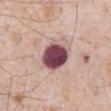follow-up = catalogued during a skin exam; not biopsied
subject = male, aged 78 to 82
image = ~15 mm crop, total-body skin-cancer survey
site = the abdomen